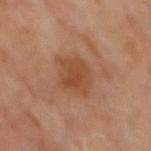Captured during whole-body skin photography for melanoma surveillance; the lesion was not biopsied.
A male patient approximately 70 years of age.
The lesion is on the mid back.
The total-body-photography lesion software estimated an area of roughly 9.5 mm², a shape eccentricity near 0.55, and a shape-asymmetry score of about 0.25 (0 = symmetric). And it measured peripheral color asymmetry of about 1.5. It also reported a classifier nevus-likeness of about 25/100 and lesion-presence confidence of about 100/100.
About 3.5 mm across.
A close-up tile cropped from a whole-body skin photograph, about 15 mm across.